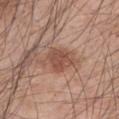image: total-body-photography crop, ~15 mm field of view
size: ~3.5 mm (longest diameter)
subject: male, aged 43 to 47
body site: the left upper arm
tile lighting: white-light illumination